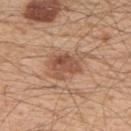Clinical impression:
The lesion was photographed on a routine skin check and not biopsied; there is no pathology result.
Image and clinical context:
The patient is a male aged 53 to 57. A 15 mm close-up extracted from a 3D total-body photography capture. On the back. An algorithmic analysis of the crop reported a mean CIELAB color near L≈53 a*≈21 b*≈31 and a normalized lesion–skin contrast near 7. And it measured a classifier nevus-likeness of about 90/100 and lesion-presence confidence of about 100/100. Measured at roughly 5.5 mm in maximum diameter.